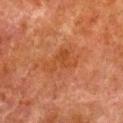Part of a total-body skin-imaging series; this lesion was reviewed on a skin check and was not flagged for biopsy.
The lesion is on the right lower leg.
A male subject, roughly 80 years of age.
A lesion tile, about 15 mm wide, cut from a 3D total-body photograph.